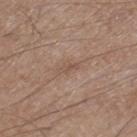notes: imaged on a skin check; not biopsied | patient: male, aged 63–67 | size: about 2.5 mm | site: the left thigh | image source: ~15 mm crop, total-body skin-cancer survey | tile lighting: white-light.